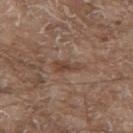workup: total-body-photography surveillance lesion; no biopsy.